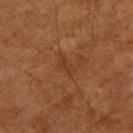Assessment: Recorded during total-body skin imaging; not selected for excision or biopsy. Acquisition and patient details: The total-body-photography lesion software estimated an area of roughly 3 mm², a shape eccentricity near 0.9, and a symmetry-axis asymmetry near 0.3. It also reported an average lesion color of about L≈34 a*≈21 b*≈31 (CIELAB), a lesion–skin lightness drop of about 5, and a normalized lesion–skin contrast near 5. Longest diameter approximately 2.5 mm. The tile uses cross-polarized illumination. On the upper back. Cropped from a total-body skin-imaging series; the visible field is about 15 mm. A male subject aged around 60.